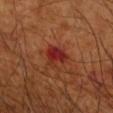Recorded during total-body skin imaging; not selected for excision or biopsy.
The lesion is located on the arm.
This image is a 15 mm lesion crop taken from a total-body photograph.
A male patient aged around 65.